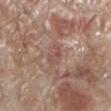Case summary:
– biopsy status: catalogued during a skin exam; not biopsied
– diameter: ≈3 mm
– patient: male, aged 68–72
– site: the left lower leg
– acquisition: total-body-photography crop, ~15 mm field of view
– TBP lesion metrics: a lesion area of about 3 mm², an outline eccentricity of about 0.9 (0 = round, 1 = elongated), and a symmetry-axis asymmetry near 0.5; a mean CIELAB color near L≈51 a*≈21 b*≈23 and a normalized lesion–skin contrast near 5; a classifier nevus-likeness of about 0/100